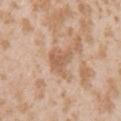About 3.5 mm across.
From the left upper arm.
A 15 mm close-up tile from a total-body photography series done for melanoma screening.
The patient is a female aged 23–27.
Captured under white-light illumination.